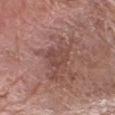{
  "image": {
    "source": "total-body photography crop",
    "field_of_view_mm": 15
  },
  "automated_metrics": {
    "area_mm2_approx": 6.0,
    "eccentricity": 0.55,
    "shape_asymmetry": 0.3,
    "nevus_likeness_0_100": 0,
    "lesion_detection_confidence_0_100": 95
  },
  "patient": {
    "sex": "female",
    "age_approx": 60
  },
  "lighting": "white-light",
  "lesion_size": {
    "long_diameter_mm_approx": 3.0
  },
  "site": "right forearm"
}